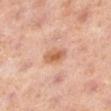Clinical impression: Captured during whole-body skin photography for melanoma surveillance; the lesion was not biopsied. Image and clinical context: On the leg. A female subject about 40 years old. A 15 mm close-up extracted from a 3D total-body photography capture. Approximately 3 mm at its widest.